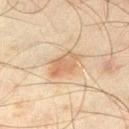follow-up: catalogued during a skin exam; not biopsied
subject: male, aged 43 to 47
automated metrics: a lesion color around L≈53 a*≈16 b*≈30 in CIELAB, roughly 8 lightness units darker than nearby skin, and a lesion-to-skin contrast of about 6 (normalized; higher = more distinct); an automated nevus-likeness rating near 95 out of 100
lesion diameter: ≈4.5 mm
imaging modality: 15 mm crop, total-body photography
site: the right thigh